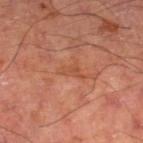• notes · total-body-photography surveillance lesion; no biopsy
• automated metrics · a footprint of about 3.5 mm², an eccentricity of roughly 0.85, and two-axis asymmetry of about 0.45; a lesion color around L≈51 a*≈27 b*≈35 in CIELAB, about 6 CIELAB-L* units darker than the surrounding skin, and a lesion-to-skin contrast of about 5 (normalized; higher = more distinct)
• illumination · cross-polarized illumination
• lesion diameter · about 3.5 mm
• site · the right lower leg
• image · ~15 mm crop, total-body skin-cancer survey
• subject · male, aged 63–67